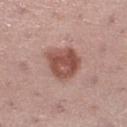This lesion was catalogued during total-body skin photography and was not selected for biopsy.
A female patient, about 55 years old.
A 15 mm close-up extracted from a 3D total-body photography capture.
On the left lower leg.
The tile uses white-light illumination.
About 4 mm across.